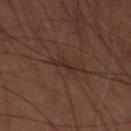Assessment:
The lesion was tiled from a total-body skin photograph and was not biopsied.
Context:
The lesion is on the leg. Cropped from a whole-body photographic skin survey; the tile spans about 15 mm. A male subject, approximately 60 years of age. About 3 mm across.